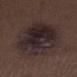<record>
  <biopsy_status>not biopsied; imaged during a skin examination</biopsy_status>
  <lesion_size>
    <long_diameter_mm_approx>10.5</long_diameter_mm_approx>
  </lesion_size>
  <site>right lower leg</site>
  <automated_metrics>
    <area_mm2_approx>45.0</area_mm2_approx>
    <eccentricity>0.8</eccentricity>
    <shape_asymmetry>0.15</shape_asymmetry>
    <vs_skin_contrast_norm>11.0</vs_skin_contrast_norm>
    <nevus_likeness_0_100>65</nevus_likeness_0_100>
    <lesion_detection_confidence_0_100>100</lesion_detection_confidence_0_100>
  </automated_metrics>
  <patient>
    <sex>male</sex>
    <age_approx>70</age_approx>
  </patient>
  <image>
    <source>total-body photography crop</source>
    <field_of_view_mm>15</field_of_view_mm>
  </image>
  <lighting>white-light</lighting>
</record>